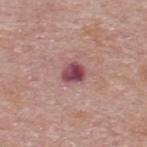{"biopsy_status": "not biopsied; imaged during a skin examination", "patient": {"sex": "male", "age_approx": 55}, "lesion_size": {"long_diameter_mm_approx": 2.5}, "site": "upper back", "image": {"source": "total-body photography crop", "field_of_view_mm": 15}}